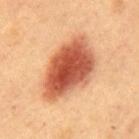Q: Was a biopsy performed?
A: no biopsy performed (imaged during a skin exam)
Q: What lighting was used for the tile?
A: cross-polarized illumination
Q: How was this image acquired?
A: 15 mm crop, total-body photography
Q: What are the patient's age and sex?
A: female, roughly 60 years of age
Q: What is the anatomic site?
A: the back
Q: What is the lesion's diameter?
A: about 8.5 mm